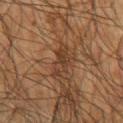Notes:
* image · total-body-photography crop, ~15 mm field of view
* location · the right upper arm
* lesion diameter · ≈3.5 mm
* subject · male, aged around 75